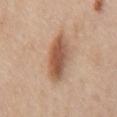This lesion was catalogued during total-body skin photography and was not selected for biopsy. The subject is a male aged approximately 60. Located on the mid back. This image is a 15 mm lesion crop taken from a total-body photograph. Approximately 5 mm at its widest.